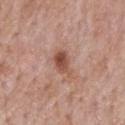{
  "biopsy_status": "not biopsied; imaged during a skin examination",
  "patient": {
    "sex": "male",
    "age_approx": 55
  },
  "lesion_size": {
    "long_diameter_mm_approx": 3.5
  },
  "image": {
    "source": "total-body photography crop",
    "field_of_view_mm": 15
  },
  "automated_metrics": {
    "shape_asymmetry": 0.3,
    "cielab_L": 50,
    "cielab_a": 23,
    "cielab_b": 28,
    "border_irregularity_0_10": 3.5,
    "peripheral_color_asymmetry": 2.0,
    "nevus_likeness_0_100": 80,
    "lesion_detection_confidence_0_100": 100
  },
  "site": "mid back"
}